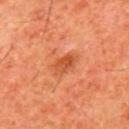{
  "biopsy_status": "not biopsied; imaged during a skin examination",
  "site": "back",
  "patient": {
    "sex": "male",
    "age_approx": 60
  },
  "automated_metrics": {
    "area_mm2_approx": 5.5,
    "eccentricity": 0.8,
    "shape_asymmetry": 0.25
  },
  "image": {
    "source": "total-body photography crop",
    "field_of_view_mm": 15
  },
  "lesion_size": {
    "long_diameter_mm_approx": 3.0
  },
  "lighting": "cross-polarized"
}